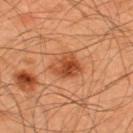Q: Was a biopsy performed?
A: imaged on a skin check; not biopsied
Q: Patient demographics?
A: male, aged around 45
Q: Lesion size?
A: about 3.5 mm
Q: What is the imaging modality?
A: ~15 mm tile from a whole-body skin photo
Q: Where on the body is the lesion?
A: the upper back